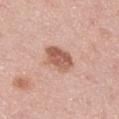Impression:
This lesion was catalogued during total-body skin photography and was not selected for biopsy.
Acquisition and patient details:
This image is a 15 mm lesion crop taken from a total-body photograph. Automated tile analysis of the lesion measured a mean CIELAB color near L≈58 a*≈23 b*≈29, roughly 13 lightness units darker than nearby skin, and a normalized border contrast of about 8.5. The software also gave a border-irregularity rating of about 1.5/10, a color-variation rating of about 4/10, and peripheral color asymmetry of about 1.5. The software also gave a lesion-detection confidence of about 100/100. The subject is a male in their mid- to late 40s. From the arm. The recorded lesion diameter is about 4 mm.